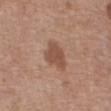Assessment: This lesion was catalogued during total-body skin photography and was not selected for biopsy. Background: A female patient, aged 73–77. Measured at roughly 4 mm in maximum diameter. The lesion is located on the abdomen. Automated image analysis of the tile measured a lesion area of about 8 mm², an outline eccentricity of about 0.75 (0 = round, 1 = elongated), and a shape-asymmetry score of about 0.3 (0 = symmetric). The software also gave a detector confidence of about 100 out of 100 that the crop contains a lesion. A close-up tile cropped from a whole-body skin photograph, about 15 mm across. Imaged with white-light lighting.